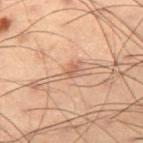Case summary:
• biopsy status · total-body-photography surveillance lesion; no biopsy
• patient · male, roughly 55 years of age
• location · the left thigh
• acquisition · ~15 mm tile from a whole-body skin photo